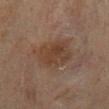Captured during whole-body skin photography for melanoma surveillance; the lesion was not biopsied. The total-body-photography lesion software estimated roughly 6 lightness units darker than nearby skin and a lesion-to-skin contrast of about 7 (normalized; higher = more distinct). The software also gave a nevus-likeness score of about 0/100 and a lesion-detection confidence of about 100/100. About 5 mm across. A 15 mm close-up extracted from a 3D total-body photography capture. The lesion is on the mid back. A male patient, aged 43–47. Imaged with cross-polarized lighting.